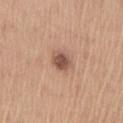<lesion>
  <biopsy_status>not biopsied; imaged during a skin examination</biopsy_status>
  <lighting>white-light</lighting>
  <image>
    <source>total-body photography crop</source>
    <field_of_view_mm>15</field_of_view_mm>
  </image>
  <lesion_size>
    <long_diameter_mm_approx>3.0</long_diameter_mm_approx>
  </lesion_size>
  <patient>
    <sex>male</sex>
    <age_approx>65</age_approx>
  </patient>
  <site>chest</site>
</lesion>